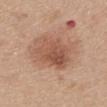No biopsy was performed on this lesion — it was imaged during a full skin examination and was not determined to be concerning.
A male subject approximately 65 years of age.
Located on the upper back.
A lesion tile, about 15 mm wide, cut from a 3D total-body photograph.
The total-body-photography lesion software estimated a footprint of about 15 mm², an outline eccentricity of about 0.45 (0 = round, 1 = elongated), and two-axis asymmetry of about 0.2. The analysis additionally found roughly 10 lightness units darker than nearby skin and a lesion-to-skin contrast of about 7 (normalized; higher = more distinct). The software also gave a nevus-likeness score of about 45/100 and a detector confidence of about 100 out of 100 that the crop contains a lesion.
The tile uses white-light illumination.